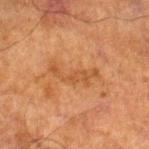{"biopsy_status": "not biopsied; imaged during a skin examination", "image": {"source": "total-body photography crop", "field_of_view_mm": 15}, "lighting": "cross-polarized", "patient": {"sex": "male", "age_approx": 70}, "automated_metrics": {"nevus_likeness_0_100": 0}, "site": "right lower leg"}